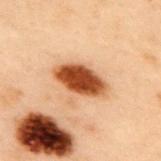| field | value |
|---|---|
| biopsy status | no biopsy performed (imaged during a skin exam) |
| body site | the back |
| diameter | ≈5 mm |
| automated lesion analysis | an area of roughly 14 mm² and a shape eccentricity near 0.8; a nevus-likeness score of about 100/100 |
| acquisition | ~15 mm crop, total-body skin-cancer survey |
| subject | male, aged approximately 55 |
| tile lighting | cross-polarized illumination |